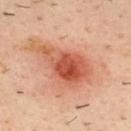Findings:
- notes: imaged on a skin check; not biopsied
- patient: male, aged around 40
- imaging modality: total-body-photography crop, ~15 mm field of view
- site: the upper back